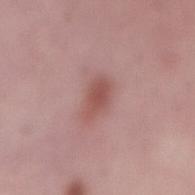Findings:
• body site · the abdomen
• diameter · ≈3.5 mm
• subject · female, in their 50s
• image source · ~15 mm crop, total-body skin-cancer survey
• tile lighting · white-light
• image-analysis metrics · an area of roughly 5.5 mm², an outline eccentricity of about 0.85 (0 = round, 1 = elongated), and a shape-asymmetry score of about 0.3 (0 = symmetric)